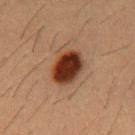Q: Was a biopsy performed?
A: no biopsy performed (imaged during a skin exam)
Q: Where on the body is the lesion?
A: the chest
Q: Automated lesion metrics?
A: about 18 CIELAB-L* units darker than the surrounding skin and a normalized border contrast of about 15.5
Q: Patient demographics?
A: male, aged approximately 55
Q: What is the imaging modality?
A: 15 mm crop, total-body photography
Q: What is the lesion's diameter?
A: ≈4.5 mm